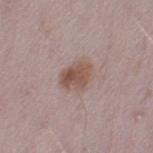notes = imaged on a skin check; not biopsied
subject = female, in their 30s
image-analysis metrics = an area of roughly 8.5 mm² and two-axis asymmetry of about 0.15; a mean CIELAB color near L≈52 a*≈18 b*≈23 and a normalized lesion–skin contrast near 8; a border-irregularity index near 1.5/10, a color-variation rating of about 4.5/10, and peripheral color asymmetry of about 1.5
lighting = white-light
size = ≈3.5 mm
image source = 15 mm crop, total-body photography
site = the left thigh